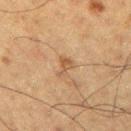notes: total-body-photography surveillance lesion; no biopsy | illumination: cross-polarized illumination | acquisition: 15 mm crop, total-body photography | lesion size: ~2.5 mm (longest diameter) | patient: male, approximately 60 years of age | image-analysis metrics: an area of roughly 3 mm², an eccentricity of roughly 0.8, and a shape-asymmetry score of about 0.55 (0 = symmetric); an automated nevus-likeness rating near 0 out of 100 and a detector confidence of about 100 out of 100 that the crop contains a lesion | location: the right upper arm.